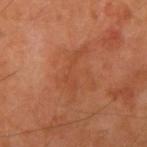biopsy_status: not biopsied; imaged during a skin examination
patient:
  sex: male
  age_approx: 65
lesion_size:
  long_diameter_mm_approx: 6.0
site: right upper arm
lighting: cross-polarized
image:
  source: total-body photography crop
  field_of_view_mm: 15
automated_metrics:
  cielab_L: 45
  cielab_a: 26
  cielab_b: 35
  vs_skin_darker_L: 5.0
  vs_skin_contrast_norm: 4.5
  border_irregularity_0_10: 10.0
  peripheral_color_asymmetry: 0.5
  nevus_likeness_0_100: 0
  lesion_detection_confidence_0_100: 100